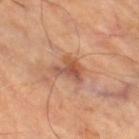follow-up: no biopsy performed (imaged during a skin exam).